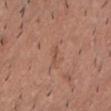This lesion was catalogued during total-body skin photography and was not selected for biopsy. This is a white-light tile. A male patient, aged approximately 40. Approximately 2.5 mm at its widest. A lesion tile, about 15 mm wide, cut from a 3D total-body photograph. Located on the chest.